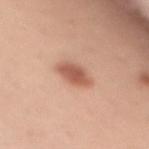follow-up = total-body-photography surveillance lesion; no biopsy | location = the abdomen | image = total-body-photography crop, ~15 mm field of view | tile lighting = white-light illumination | diameter = ~5 mm (longest diameter) | patient = female, aged approximately 35.